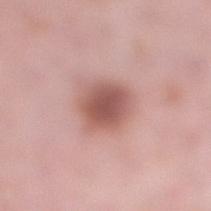The lesion was photographed on a routine skin check and not biopsied; there is no pathology result. A lesion tile, about 15 mm wide, cut from a 3D total-body photograph. Located on the right lower leg. Longest diameter approximately 4 mm. The total-body-photography lesion software estimated a lesion color around L≈55 a*≈23 b*≈24 in CIELAB, roughly 13 lightness units darker than nearby skin, and a normalized border contrast of about 9. And it measured a border-irregularity index near 1.5/10 and internal color variation of about 3.5 on a 0–10 scale. The patient is a female approximately 40 years of age. Imaged with white-light lighting.